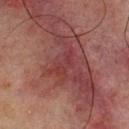This lesion was catalogued during total-body skin photography and was not selected for biopsy.
Cropped from a whole-body photographic skin survey; the tile spans about 15 mm.
The recorded lesion diameter is about 3.5 mm.
A male subject, roughly 65 years of age.
This is a cross-polarized tile.
From the back.